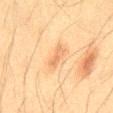Image and clinical context: A close-up tile cropped from a whole-body skin photograph, about 15 mm across. This is a cross-polarized tile. The lesion is located on the front of the torso. Measured at roughly 3 mm in maximum diameter. Automated image analysis of the tile measured a footprint of about 3.5 mm² and an outline eccentricity of about 0.9 (0 = round, 1 = elongated). And it measured an average lesion color of about L≈55 a*≈18 b*≈34 (CIELAB), roughly 7 lightness units darker than nearby skin, and a lesion-to-skin contrast of about 5 (normalized; higher = more distinct). The analysis additionally found border irregularity of about 4 on a 0–10 scale, a within-lesion color-variation index near 0/10, and a peripheral color-asymmetry measure near 0. The patient is a male aged around 35.